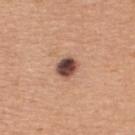- workup — imaged on a skin check; not biopsied
- site — the back
- image-analysis metrics — a lesion color around L≈47 a*≈21 b*≈25 in CIELAB, a lesion–skin lightness drop of about 19, and a normalized border contrast of about 13.5; a border-irregularity index near 1.5/10 and radial color variation of about 2; a nevus-likeness score of about 70/100 and a lesion-detection confidence of about 100/100
- image source — ~15 mm tile from a whole-body skin photo
- lighting — white-light
- patient — female, roughly 30 years of age